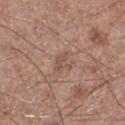  biopsy_status: not biopsied; imaged during a skin examination
  lesion_size:
    long_diameter_mm_approx: 2.5
  patient:
    sex: male
    age_approx: 65
  site: left lower leg
  automated_metrics:
    cielab_L: 52
    cielab_a: 19
    cielab_b: 25
    vs_skin_darker_L: 7.0
    vs_skin_contrast_norm: 5.0
  image:
    source: total-body photography crop
    field_of_view_mm: 15
  lighting: white-light